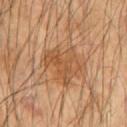Q: Was this lesion biopsied?
A: no biopsy performed (imaged during a skin exam)
Q: What is the anatomic site?
A: the right upper arm
Q: What is the imaging modality?
A: total-body-photography crop, ~15 mm field of view
Q: What are the patient's age and sex?
A: male, approximately 60 years of age
Q: What lighting was used for the tile?
A: cross-polarized
Q: What is the lesion's diameter?
A: about 5 mm